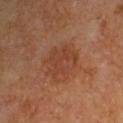Findings:
– biopsy status — catalogued during a skin exam; not biopsied
– lesion diameter — ~5 mm (longest diameter)
– lighting — cross-polarized
– body site — the left upper arm
– image — ~15 mm crop, total-body skin-cancer survey
– patient — male, about 65 years old